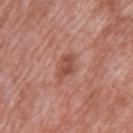Q: Was this lesion biopsied?
A: catalogued during a skin exam; not biopsied